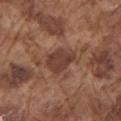| field | value |
|---|---|
| notes | total-body-photography surveillance lesion; no biopsy |
| body site | the left upper arm |
| imaging modality | 15 mm crop, total-body photography |
| patient | male, aged 73–77 |
| lesion diameter | about 4 mm |